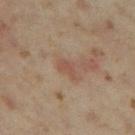Findings:
– follow-up — imaged on a skin check; not biopsied
– site — the left thigh
– automated metrics — an area of roughly 2.5 mm², an eccentricity of roughly 0.85, and a symmetry-axis asymmetry near 0.4; a mean CIELAB color near L≈48 a*≈19 b*≈26, a lesion–skin lightness drop of about 7, and a normalized lesion–skin contrast near 5.5; a nevus-likeness score of about 0/100
– subject — female, in their mid-30s
– diameter — ≈2.5 mm
– illumination — cross-polarized illumination
– image — ~15 mm crop, total-body skin-cancer survey